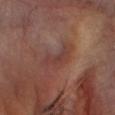{"biopsy_status": "not biopsied; imaged during a skin examination", "patient": {"sex": "male", "age_approx": 70}, "image": {"source": "total-body photography crop", "field_of_view_mm": 15}, "site": "head or neck"}